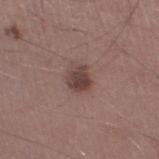No biopsy was performed on this lesion — it was imaged during a full skin examination and was not determined to be concerning. The patient is a male aged approximately 45. The lesion's longest dimension is about 2.5 mm. Located on the left thigh. Imaged with white-light lighting. Cropped from a whole-body photographic skin survey; the tile spans about 15 mm.